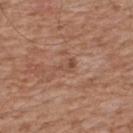Q: Was a biopsy performed?
A: total-body-photography surveillance lesion; no biopsy
Q: What is the imaging modality?
A: ~15 mm tile from a whole-body skin photo
Q: What are the patient's age and sex?
A: male, aged around 65
Q: Lesion location?
A: the back